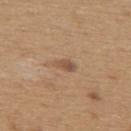Q: How was this image acquired?
A: total-body-photography crop, ~15 mm field of view
Q: What is the anatomic site?
A: the upper back
Q: Who is the patient?
A: male, in their mid- to late 60s
Q: Automated lesion metrics?
A: a lesion area of about 3 mm², a shape eccentricity near 0.85, and a symmetry-axis asymmetry near 0.35; an average lesion color of about L≈52 a*≈18 b*≈31 (CIELAB) and roughly 9 lightness units darker than nearby skin; border irregularity of about 3 on a 0–10 scale and internal color variation of about 1.5 on a 0–10 scale
Q: What lighting was used for the tile?
A: white-light illumination
Q: What is the lesion's diameter?
A: about 2.5 mm